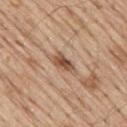Notes:
- biopsy status — catalogued during a skin exam; not biopsied
- patient — male, aged approximately 70
- lighting — white-light
- site — the back
- size — ≈3.5 mm
- image source — 15 mm crop, total-body photography